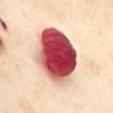| field | value |
|---|---|
| notes | imaged on a skin check; not biopsied |
| diameter | about 7.5 mm |
| image source | ~15 mm crop, total-body skin-cancer survey |
| site | the abdomen |
| patient | female, aged 53 to 57 |